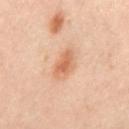Findings:
* notes · catalogued during a skin exam; not biopsied
* image source · 15 mm crop, total-body photography
* patient · male, in their mid-60s
* automated metrics · a mean CIELAB color near L≈65 a*≈24 b*≈36 and a normalized lesion–skin contrast near 7; a border-irregularity index near 2.5/10 and a within-lesion color-variation index near 4/10
* lesion size · ~4 mm (longest diameter)
* illumination · cross-polarized illumination
* site · the mid back